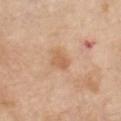This lesion was catalogued during total-body skin photography and was not selected for biopsy.
From the chest.
A 15 mm crop from a total-body photograph taken for skin-cancer surveillance.
The patient is a female approximately 65 years of age.